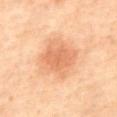notes: no biopsy performed (imaged during a skin exam) | automated metrics: a lesion-to-skin contrast of about 5.5 (normalized; higher = more distinct); a border-irregularity rating of about 2/10 and radial color variation of about 1; a classifier nevus-likeness of about 70/100 and lesion-presence confidence of about 100/100 | location: the front of the torso | patient: male, aged around 70 | image source: ~15 mm crop, total-body skin-cancer survey.